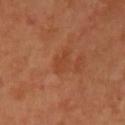{"biopsy_status": "not biopsied; imaged during a skin examination"}